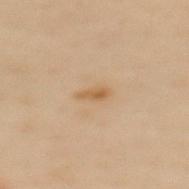The lesion was tiled from a total-body skin photograph and was not biopsied. A region of skin cropped from a whole-body photographic capture, roughly 15 mm wide. Imaged with cross-polarized lighting. Approximately 2.5 mm at its widest. The subject is a female aged 58 to 62. Automated image analysis of the tile measured a shape eccentricity near 0.9 and a symmetry-axis asymmetry near 0.4. And it measured a normalized lesion–skin contrast near 7. The lesion is located on the upper back.